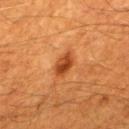follow-up: no biopsy performed (imaged during a skin exam)
patient: male, roughly 60 years of age
acquisition: ~15 mm tile from a whole-body skin photo
TBP lesion metrics: an average lesion color of about L≈44 a*≈30 b*≈41 (CIELAB) and about 12 CIELAB-L* units darker than the surrounding skin; a border-irregularity rating of about 2/10, internal color variation of about 4.5 on a 0–10 scale, and a peripheral color-asymmetry measure near 1.5
lighting: cross-polarized
anatomic site: the mid back